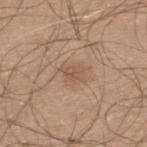This lesion was catalogued during total-body skin photography and was not selected for biopsy. Located on the upper back. The subject is a male about 45 years old. About 2.5 mm across. A region of skin cropped from a whole-body photographic capture, roughly 15 mm wide.